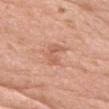Impression:
No biopsy was performed on this lesion — it was imaged during a full skin examination and was not determined to be concerning.
Context:
About 3 mm across. The lesion is on the chest. The patient is a female aged approximately 70. A close-up tile cropped from a whole-body skin photograph, about 15 mm across.